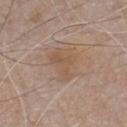Q: Lesion location?
A: the front of the torso
Q: What is the lesion's diameter?
A: ~4.5 mm (longest diameter)
Q: Patient demographics?
A: male, roughly 75 years of age
Q: How was this image acquired?
A: ~15 mm tile from a whole-body skin photo